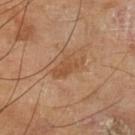Recorded during total-body skin imaging; not selected for excision or biopsy.
The lesion's longest dimension is about 4 mm.
This image is a 15 mm lesion crop taken from a total-body photograph.
The lesion is on the leg.
The patient is a male in their mid-60s.
The tile uses cross-polarized illumination.
An algorithmic analysis of the crop reported a lesion area of about 6 mm², an eccentricity of roughly 0.9, and a shape-asymmetry score of about 0.35 (0 = symmetric). The analysis additionally found a mean CIELAB color near L≈51 a*≈21 b*≈35, a lesion–skin lightness drop of about 8, and a lesion-to-skin contrast of about 6 (normalized; higher = more distinct). The analysis additionally found an automated nevus-likeness rating near 0 out of 100.